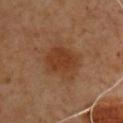biopsy status: imaged on a skin check; not biopsied
size: about 5 mm
image-analysis metrics: a footprint of about 17 mm²; a nevus-likeness score of about 75/100 and lesion-presence confidence of about 100/100
image source: ~15 mm crop, total-body skin-cancer survey
subject: male, aged 48 to 52
location: the chest
tile lighting: cross-polarized